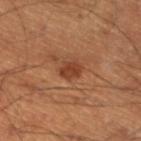Impression:
Captured during whole-body skin photography for melanoma surveillance; the lesion was not biopsied.
Background:
From the left lower leg. Imaged with cross-polarized lighting. The patient is a male aged 48 to 52. A 15 mm close-up tile from a total-body photography series done for melanoma screening.